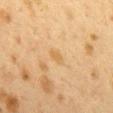Assessment: This lesion was catalogued during total-body skin photography and was not selected for biopsy. Acquisition and patient details: A female patient, about 40 years old. The lesion-visualizer software estimated an area of roughly 3 mm², a shape eccentricity near 0.85, and a shape-asymmetry score of about 0.3 (0 = symmetric). It also reported roughly 5 lightness units darker than nearby skin and a normalized lesion–skin contrast near 5. The analysis additionally found border irregularity of about 2.5 on a 0–10 scale and a peripheral color-asymmetry measure near 0. The analysis additionally found a lesion-detection confidence of about 100/100. Measured at roughly 2.5 mm in maximum diameter. The lesion is on the mid back. Cropped from a total-body skin-imaging series; the visible field is about 15 mm. The tile uses cross-polarized illumination.